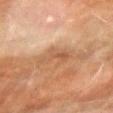| field | value |
|---|---|
| notes | imaged on a skin check; not biopsied |
| site | the left forearm |
| patient | male, aged approximately 70 |
| imaging modality | ~15 mm crop, total-body skin-cancer survey |